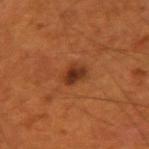Clinical impression:
This lesion was catalogued during total-body skin photography and was not selected for biopsy.
Acquisition and patient details:
The subject is a male approximately 55 years of age. On the arm. About 2.5 mm across. Cropped from a whole-body photographic skin survey; the tile spans about 15 mm.